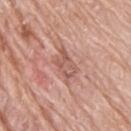lighting: white-light
image:
  source: total-body photography crop
  field_of_view_mm: 15
site: back
automated_metrics:
  nevus_likeness_0_100: 0
  lesion_detection_confidence_0_100: 75
patient:
  sex: male
  age_approx: 75
lesion_size:
  long_diameter_mm_approx: 4.0